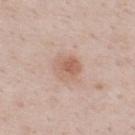workup: total-body-photography surveillance lesion; no biopsy | tile lighting: white-light illumination | site: the upper back | image source: 15 mm crop, total-body photography | subject: male, aged 48 to 52 | lesion diameter: ≈3.5 mm | automated lesion analysis: a border-irregularity index near 1.5/10, a within-lesion color-variation index near 4.5/10, and radial color variation of about 1.5.